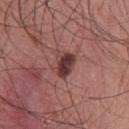* notes · catalogued during a skin exam; not biopsied
* lesion size · ~3.5 mm (longest diameter)
* illumination · white-light illumination
* acquisition · ~15 mm tile from a whole-body skin photo
* site · the chest
* patient · male, approximately 40 years of age